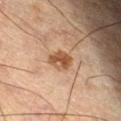Measured at roughly 2.5 mm in maximum diameter.
Located on the left thigh.
The subject is a male aged 63–67.
The tile uses cross-polarized illumination.
This image is a 15 mm lesion crop taken from a total-body photograph.